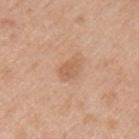<record>
  <biopsy_status>not biopsied; imaged during a skin examination</biopsy_status>
  <site>arm</site>
  <lighting>white-light</lighting>
  <lesion_size>
    <long_diameter_mm_approx>3.0</long_diameter_mm_approx>
  </lesion_size>
  <patient>
    <sex>male</sex>
    <age_approx>45</age_approx>
  </patient>
  <image>
    <source>total-body photography crop</source>
    <field_of_view_mm>15</field_of_view_mm>
  </image>
</record>